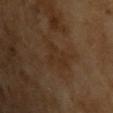{"biopsy_status": "not biopsied; imaged during a skin examination", "lesion_size": {"long_diameter_mm_approx": 4.0}, "lighting": "cross-polarized", "patient": {"sex": "male", "age_approx": 65}, "image": {"source": "total-body photography crop", "field_of_view_mm": 15}}